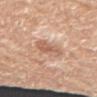Automated tile analysis of the lesion measured an average lesion color of about L≈61 a*≈21 b*≈31 (CIELAB) and roughly 10 lightness units darker than nearby skin. It also reported an automated nevus-likeness rating near 15 out of 100 and lesion-presence confidence of about 100/100. A 15 mm close-up tile from a total-body photography series done for melanoma screening. The lesion is on the arm. A female subject in their mid-70s. Approximately 3 mm at its widest. The tile uses white-light illumination.